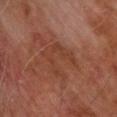Image and clinical context: Longest diameter approximately 5.5 mm. This image is a 15 mm lesion crop taken from a total-body photograph. From the chest. A male subject, aged 58–62. The total-body-photography lesion software estimated a lesion area of about 12 mm², an outline eccentricity of about 0.6 (0 = round, 1 = elongated), and a symmetry-axis asymmetry near 0.6. The analysis additionally found a classifier nevus-likeness of about 0/100 and lesion-presence confidence of about 85/100. Imaged with cross-polarized lighting.